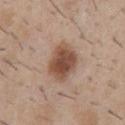notes = total-body-photography surveillance lesion; no biopsy
subject = male, aged around 30
site = the chest
acquisition = ~15 mm tile from a whole-body skin photo